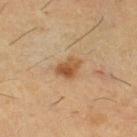Captured during whole-body skin photography for melanoma surveillance; the lesion was not biopsied. The recorded lesion diameter is about 3 mm. A male subject approximately 60 years of age. Imaged with cross-polarized lighting. The total-body-photography lesion software estimated a footprint of about 5.5 mm², a shape eccentricity near 0.65, and a symmetry-axis asymmetry near 0.25. And it measured a border-irregularity rating of about 2.5/10 and peripheral color asymmetry of about 2. The analysis additionally found an automated nevus-likeness rating near 95 out of 100. On the right thigh. A 15 mm close-up extracted from a 3D total-body photography capture.